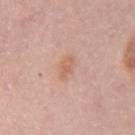follow-up: imaged on a skin check; not biopsied
automated metrics: a lesion area of about 3.5 mm², a shape eccentricity near 0.75, and a shape-asymmetry score of about 0.2 (0 = symmetric); an average lesion color of about L≈62 a*≈21 b*≈30 (CIELAB) and a normalized border contrast of about 5.5; a border-irregularity index near 2/10, a within-lesion color-variation index near 1.5/10, and radial color variation of about 0.5; a detector confidence of about 100 out of 100 that the crop contains a lesion
site: the left upper arm
acquisition: ~15 mm crop, total-body skin-cancer survey
patient: female, in their 60s
lesion diameter: ~2.5 mm (longest diameter)